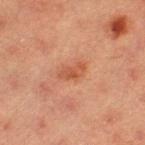No biopsy was performed on this lesion — it was imaged during a full skin examination and was not determined to be concerning.
The lesion is on the left thigh.
Measured at roughly 3 mm in maximum diameter.
A 15 mm crop from a total-body photograph taken for skin-cancer surveillance.
Imaged with cross-polarized lighting.
A female patient, about 40 years old.